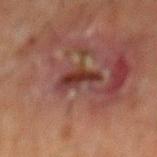This lesion was catalogued during total-body skin photography and was not selected for biopsy. This is a cross-polarized tile. A male subject, aged 58–62. The recorded lesion diameter is about 4 mm. A close-up tile cropped from a whole-body skin photograph, about 15 mm across. The total-body-photography lesion software estimated a mean CIELAB color near L≈26 a*≈20 b*≈20 and about 9 CIELAB-L* units darker than the surrounding skin. The analysis additionally found border irregularity of about 4.5 on a 0–10 scale and a peripheral color-asymmetry measure near 1. On the mid back.